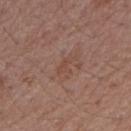Captured during whole-body skin photography for melanoma surveillance; the lesion was not biopsied.
Cropped from a total-body skin-imaging series; the visible field is about 15 mm.
Automated tile analysis of the lesion measured an eccentricity of roughly 0.85 and two-axis asymmetry of about 0.55. And it measured an average lesion color of about L≈46 a*≈20 b*≈26 (CIELAB) and a normalized lesion–skin contrast near 5. It also reported a border-irregularity index near 6.5/10.
Captured under white-light illumination.
A female subject, in their mid-50s.
From the right upper arm.
Approximately 3 mm at its widest.